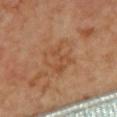Clinical summary: The tile uses cross-polarized illumination. The subject is female. Longest diameter approximately 4 mm. Cropped from a whole-body photographic skin survey; the tile spans about 15 mm. The lesion is located on the upper back.